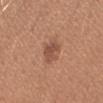| feature | finding |
|---|---|
| notes | no biopsy performed (imaged during a skin exam) |
| subject | female, aged 18–22 |
| acquisition | ~15 mm tile from a whole-body skin photo |
| tile lighting | white-light |
| location | the arm |